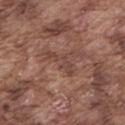No biopsy was performed on this lesion — it was imaged during a full skin examination and was not determined to be concerning. The total-body-photography lesion software estimated a border-irregularity rating of about 8/10, internal color variation of about 0.5 on a 0–10 scale, and a peripheral color-asymmetry measure near 0. And it measured a classifier nevus-likeness of about 0/100 and a lesion-detection confidence of about 80/100. Captured under white-light illumination. A male patient aged 73 to 77. Cropped from a total-body skin-imaging series; the visible field is about 15 mm. The lesion's longest dimension is about 4 mm. On the mid back.